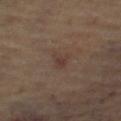Clinical impression:
Part of a total-body skin-imaging series; this lesion was reviewed on a skin check and was not flagged for biopsy.
Clinical summary:
A subject in their 60s. The lesion is located on the left thigh. About 3 mm across. This image is a 15 mm lesion crop taken from a total-body photograph. The lesion-visualizer software estimated a lesion area of about 4 mm² and a symmetry-axis asymmetry near 0.4. The analysis additionally found a mean CIELAB color near L≈37 a*≈15 b*≈21 and a lesion–skin lightness drop of about 5.